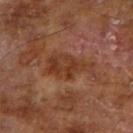Assessment:
This lesion was catalogued during total-body skin photography and was not selected for biopsy.
Clinical summary:
A 15 mm close-up extracted from a 3D total-body photography capture. The lesion is located on the right upper arm. Captured under cross-polarized illumination. A male subject, in their mid-60s.